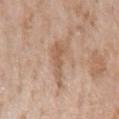Recorded during total-body skin imaging; not selected for excision or biopsy.
A female subject approximately 70 years of age.
On the left forearm.
A 15 mm crop from a total-body photograph taken for skin-cancer surveillance.